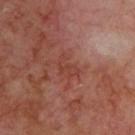notes: total-body-photography surveillance lesion; no biopsy
site: the back
patient: male, aged around 70
image source: ~15 mm tile from a whole-body skin photo